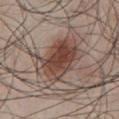Q: Was a biopsy performed?
A: imaged on a skin check; not biopsied
Q: Patient demographics?
A: male, approximately 45 years of age
Q: What is the anatomic site?
A: the chest
Q: Lesion size?
A: about 6 mm
Q: Automated lesion metrics?
A: a footprint of about 20 mm² and an eccentricity of roughly 0.75; an automated nevus-likeness rating near 100 out of 100 and a lesion-detection confidence of about 100/100
Q: How was this image acquired?
A: ~15 mm tile from a whole-body skin photo
Q: What lighting was used for the tile?
A: white-light